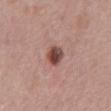| field | value |
|---|---|
| follow-up | total-body-photography surveillance lesion; no biopsy |
| imaging modality | ~15 mm tile from a whole-body skin photo |
| subject | female, aged around 65 |
| site | the mid back |
| size | about 3 mm |
| TBP lesion metrics | a footprint of about 5 mm², a shape eccentricity near 0.65, and a symmetry-axis asymmetry near 0.15; a color-variation rating of about 7/10 and peripheral color asymmetry of about 2.5 |